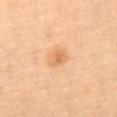Clinical summary:
The tile uses cross-polarized illumination. The patient is a female aged approximately 60. A close-up tile cropped from a whole-body skin photograph, about 15 mm across. Approximately 2.5 mm at its widest. From the abdomen.